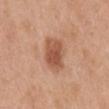Findings:
* biopsy status: imaged on a skin check; not biopsied
* TBP lesion metrics: border irregularity of about 1.5 on a 0–10 scale, a within-lesion color-variation index near 4/10, and peripheral color asymmetry of about 1
* image source: 15 mm crop, total-body photography
* lesion diameter: ~4 mm (longest diameter)
* body site: the mid back
* patient: male, about 30 years old